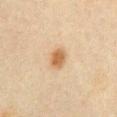Case summary:
– biopsy status · catalogued during a skin exam; not biopsied
– location · the abdomen
– patient · female, in their mid- to late 60s
– imaging modality · ~15 mm crop, total-body skin-cancer survey